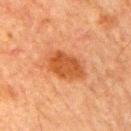The lesion was photographed on a routine skin check and not biopsied; there is no pathology result. A male patient aged 78 to 82. From the right upper arm. Cropped from a whole-body photographic skin survey; the tile spans about 15 mm.